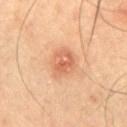follow-up = catalogued during a skin exam; not biopsied
anatomic site = the abdomen
TBP lesion metrics = a nevus-likeness score of about 10/100 and a lesion-detection confidence of about 100/100
lighting = cross-polarized
subject = male, approximately 50 years of age
image source = 15 mm crop, total-body photography